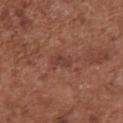workup: imaged on a skin check; not biopsied.